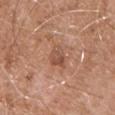workup: total-body-photography surveillance lesion; no biopsy
illumination: white-light illumination
patient: male, aged around 55
acquisition: 15 mm crop, total-body photography
location: the chest